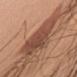<tbp_lesion>
<biopsy_status>not biopsied; imaged during a skin examination</biopsy_status>
<site>left upper arm</site>
<patient>
  <sex>male</sex>
  <age_approx>50</age_approx>
</patient>
<lighting>white-light</lighting>
<lesion_size>
  <long_diameter_mm_approx>6.5</long_diameter_mm_approx>
</lesion_size>
<image>
  <source>total-body photography crop</source>
  <field_of_view_mm>15</field_of_view_mm>
</image>
</tbp_lesion>